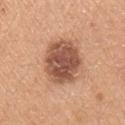Impression: Imaged during a routine full-body skin examination; the lesion was not biopsied and no histopathology is available. Context: Located on the right upper arm. The total-body-photography lesion software estimated an area of roughly 20 mm², an eccentricity of roughly 0.4, and two-axis asymmetry of about 0.1. It also reported a mean CIELAB color near L≈53 a*≈23 b*≈31 and a normalized border contrast of about 10. The software also gave border irregularity of about 1.5 on a 0–10 scale and radial color variation of about 1.5. A roughly 15 mm field-of-view crop from a total-body skin photograph. Captured under white-light illumination. A male patient aged around 20. The recorded lesion diameter is about 5.5 mm.